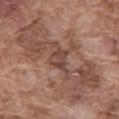| field | value |
|---|---|
| notes | no biopsy performed (imaged during a skin exam) |
| patient | male, in their mid- to late 70s |
| location | the abdomen |
| tile lighting | white-light |
| image source | ~15 mm tile from a whole-body skin photo |